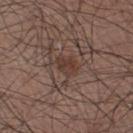biopsy status: no biopsy performed (imaged during a skin exam)
subject: male, approximately 65 years of age
image source: ~15 mm tile from a whole-body skin photo
site: the left lower leg